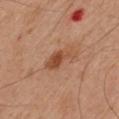Background:
The subject is a male aged around 45. Cropped from a whole-body photographic skin survey; the tile spans about 15 mm. About 4 mm across. Automated image analysis of the tile measured a lesion area of about 6.5 mm², a shape eccentricity near 0.8, and a shape-asymmetry score of about 0.4 (0 = symmetric). The software also gave an average lesion color of about L≈45 a*≈22 b*≈31 (CIELAB), roughly 9 lightness units darker than nearby skin, and a normalized lesion–skin contrast near 7.5. It also reported a border-irregularity rating of about 4/10, a within-lesion color-variation index near 4/10, and peripheral color asymmetry of about 1. The tile uses cross-polarized illumination. From the left upper arm.